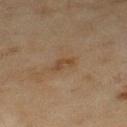{"biopsy_status": "not biopsied; imaged during a skin examination", "image": {"source": "total-body photography crop", "field_of_view_mm": 15}, "patient": {"sex": "female", "age_approx": 60}, "lesion_size": {"long_diameter_mm_approx": 2.5}, "lighting": "cross-polarized", "site": "left lower leg"}